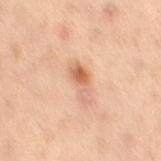{
  "biopsy_status": "not biopsied; imaged during a skin examination",
  "image": {
    "source": "total-body photography crop",
    "field_of_view_mm": 15
  },
  "patient": {
    "sex": "female",
    "age_approx": 55
  },
  "site": "lower back"
}